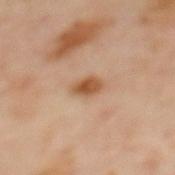{"biopsy_status": "not biopsied; imaged during a skin examination", "lesion_size": {"long_diameter_mm_approx": 3.0}, "site": "upper back", "patient": {"sex": "female", "age_approx": 60}, "lighting": "cross-polarized", "image": {"source": "total-body photography crop", "field_of_view_mm": 15}}